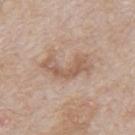Impression: No biopsy was performed on this lesion — it was imaged during a full skin examination and was not determined to be concerning. Background: This image is a 15 mm lesion crop taken from a total-body photograph. The subject is a male aged 63–67. The recorded lesion diameter is about 6 mm. On the mid back.